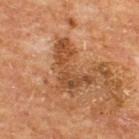Assessment: Imaged during a routine full-body skin examination; the lesion was not biopsied and no histopathology is available. Acquisition and patient details: A roughly 15 mm field-of-view crop from a total-body skin photograph. On the upper back. Captured under cross-polarized illumination. The lesion-visualizer software estimated an area of roughly 13 mm² and a shape eccentricity near 0.85. It also reported an average lesion color of about L≈43 a*≈21 b*≈34 (CIELAB) and a lesion–skin lightness drop of about 10. The software also gave peripheral color asymmetry of about 1.5. The subject is a male in their mid- to late 60s.